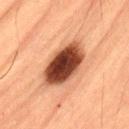  biopsy_status: not biopsied; imaged during a skin examination
  patient:
    sex: male
    age_approx: 55
  site: abdomen
  image:
    source: total-body photography crop
    field_of_view_mm: 15